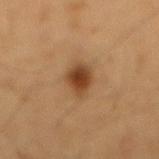An algorithmic analysis of the crop reported an area of roughly 6.5 mm², an outline eccentricity of about 0.8 (0 = round, 1 = elongated), and a shape-asymmetry score of about 0.25 (0 = symmetric). It also reported a border-irregularity rating of about 2.5/10, internal color variation of about 4 on a 0–10 scale, and a peripheral color-asymmetry measure near 1.
The lesion is located on the abdomen.
The tile uses cross-polarized illumination.
A male patient, approximately 55 years of age.
Cropped from a total-body skin-imaging series; the visible field is about 15 mm.